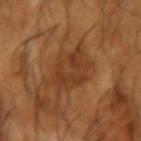{
  "biopsy_status": "not biopsied; imaged during a skin examination",
  "site": "left forearm",
  "automated_metrics": {
    "area_mm2_approx": 17.0,
    "eccentricity": 0.75,
    "cielab_L": 39,
    "cielab_a": 22,
    "cielab_b": 34,
    "vs_skin_darker_L": 7.0,
    "vs_skin_contrast_norm": 6.5,
    "border_irregularity_0_10": 4.5,
    "color_variation_0_10": 3.5,
    "peripheral_color_asymmetry": 1.0,
    "lesion_detection_confidence_0_100": 95
  },
  "image": {
    "source": "total-body photography crop",
    "field_of_view_mm": 15
  },
  "lesion_size": {
    "long_diameter_mm_approx": 6.5
  },
  "lighting": "cross-polarized",
  "patient": {
    "sex": "male",
    "age_approx": 65
  }
}